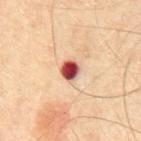biopsy_status: not biopsied; imaged during a skin examination
automated_metrics:
  area_mm2_approx: 4.5
  eccentricity: 0.45
  shape_asymmetry: 0.25
  vs_skin_darker_L: 24.0
  vs_skin_contrast_norm: 15.0
  border_irregularity_0_10: 2.0
  color_variation_0_10: 9.0
  peripheral_color_asymmetry: 2.5
  lesion_detection_confidence_0_100: 100
lighting: cross-polarized
image:
  source: total-body photography crop
  field_of_view_mm: 15
patient:
  sex: male
  age_approx: 65
site: abdomen